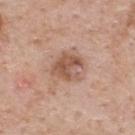Part of a total-body skin-imaging series; this lesion was reviewed on a skin check and was not flagged for biopsy. Approximately 3.5 mm at its widest. Automated image analysis of the tile measured an area of roughly 9 mm² and an outline eccentricity of about 0.5 (0 = round, 1 = elongated). And it measured a mean CIELAB color near L≈55 a*≈21 b*≈29, about 11 CIELAB-L* units darker than the surrounding skin, and a normalized lesion–skin contrast near 7.5. The software also gave a nevus-likeness score of about 10/100 and lesion-presence confidence of about 100/100. The lesion is on the upper back. The subject is a male approximately 60 years of age. A close-up tile cropped from a whole-body skin photograph, about 15 mm across. The tile uses white-light illumination.